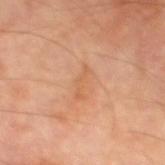Recorded during total-body skin imaging; not selected for excision or biopsy.
The total-body-photography lesion software estimated a border-irregularity rating of about 6.5/10, a color-variation rating of about 0/10, and radial color variation of about 0.
A roughly 15 mm field-of-view crop from a total-body skin photograph.
The lesion is located on the left thigh.
The patient is a male in their 70s.
The lesion's longest dimension is about 3.5 mm.
The tile uses cross-polarized illumination.